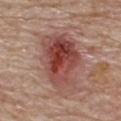Assessment: The lesion was tiled from a total-body skin photograph and was not biopsied. Background: The lesion is on the upper back. The recorded lesion diameter is about 7 mm. This is a white-light tile. The total-body-photography lesion software estimated a mean CIELAB color near L≈46 a*≈25 b*≈25, roughly 12 lightness units darker than nearby skin, and a lesion-to-skin contrast of about 9 (normalized; higher = more distinct). It also reported a border-irregularity index near 3/10 and a color-variation rating of about 9.5/10. And it measured a nevus-likeness score of about 70/100 and a lesion-detection confidence of about 100/100. A male subject, aged 78 to 82. A 15 mm close-up tile from a total-body photography series done for melanoma screening.